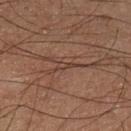Case summary:
- follow-up — total-body-photography surveillance lesion; no biopsy
- lighting — cross-polarized illumination
- TBP lesion metrics — roughly 6 lightness units darker than nearby skin and a normalized lesion–skin contrast near 6; border irregularity of about 5 on a 0–10 scale and peripheral color asymmetry of about 0
- diameter — about 2.5 mm
- site — the left lower leg
- image — 15 mm crop, total-body photography
- patient — male, about 60 years old